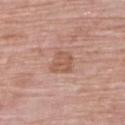Part of a total-body skin-imaging series; this lesion was reviewed on a skin check and was not flagged for biopsy. The total-body-photography lesion software estimated a lesion area of about 7 mm², a shape eccentricity near 0.4, and a symmetry-axis asymmetry near 0.3. The analysis additionally found a border-irregularity rating of about 3/10 and radial color variation of about 1. It also reported an automated nevus-likeness rating near 0 out of 100 and a lesion-detection confidence of about 100/100. The patient is a female approximately 65 years of age. This image is a 15 mm lesion crop taken from a total-body photograph. From the back. About 3 mm across. This is a white-light tile.